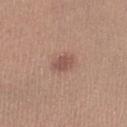notes: total-body-photography surveillance lesion; no biopsy
TBP lesion metrics: a footprint of about 4.5 mm² and an outline eccentricity of about 0.75 (0 = round, 1 = elongated); a border-irregularity index near 2/10 and a peripheral color-asymmetry measure near 0.5
site: the left lower leg
diameter: about 3 mm
subject: female, aged 43–47
acquisition: ~15 mm crop, total-body skin-cancer survey